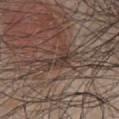No biopsy was performed on this lesion — it was imaged during a full skin examination and was not determined to be concerning.
Located on the abdomen.
A male subject, in their 50s.
Cropped from a whole-body photographic skin survey; the tile spans about 15 mm.
Longest diameter approximately 5 mm.
An algorithmic analysis of the crop reported a shape eccentricity near 0.9 and two-axis asymmetry of about 0.45. The software also gave a border-irregularity index near 6.5/10. The analysis additionally found an automated nevus-likeness rating near 0 out of 100 and a detector confidence of about 0 out of 100 that the crop contains a lesion.